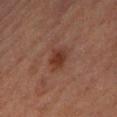Clinical impression: Captured during whole-body skin photography for melanoma surveillance; the lesion was not biopsied. Image and clinical context: This image is a 15 mm lesion crop taken from a total-body photograph. A male patient about 85 years old or older. Located on the leg.